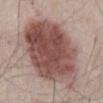The lesion was tiled from a total-body skin photograph and was not biopsied. The tile uses white-light illumination. The patient is a male roughly 65 years of age. The lesion is on the abdomen. A 15 mm close-up extracted from a 3D total-body photography capture. Approximately 10.5 mm at its widest. The total-body-photography lesion software estimated an area of roughly 60 mm², an eccentricity of roughly 0.65, and two-axis asymmetry of about 0.15. It also reported roughly 16 lightness units darker than nearby skin. The analysis additionally found a classifier nevus-likeness of about 100/100 and a lesion-detection confidence of about 100/100.